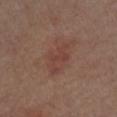Captured during whole-body skin photography for melanoma surveillance; the lesion was not biopsied. A male subject about 70 years old. A 15 mm close-up extracted from a 3D total-body photography capture. The lesion is on the front of the torso. Imaged with white-light lighting. The recorded lesion diameter is about 4 mm.